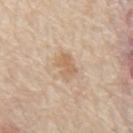follow-up: total-body-photography surveillance lesion; no biopsy | patient: male, aged around 65 | site: the abdomen | acquisition: ~15 mm tile from a whole-body skin photo | automated metrics: a lesion area of about 6 mm², a shape eccentricity near 0.75, and a symmetry-axis asymmetry near 0.2; a within-lesion color-variation index near 2/10 and radial color variation of about 1; a nevus-likeness score of about 5/100 | tile lighting: white-light.